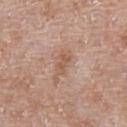Q: Was a biopsy performed?
A: no biopsy performed (imaged during a skin exam)
Q: How was the tile lit?
A: white-light illumination
Q: Patient demographics?
A: male, aged around 70
Q: How was this image acquired?
A: ~15 mm crop, total-body skin-cancer survey
Q: Where on the body is the lesion?
A: the chest